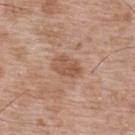notes=imaged on a skin check; not biopsied
image source=15 mm crop, total-body photography
patient=male, aged approximately 50
image-analysis metrics=an area of roughly 6 mm², an eccentricity of roughly 0.75, and a symmetry-axis asymmetry near 0.2; a lesion color around L≈54 a*≈21 b*≈31 in CIELAB; a border-irregularity rating of about 2/10 and radial color variation of about 1; a nevus-likeness score of about 0/100 and lesion-presence confidence of about 100/100
lesion size=≈3.5 mm
location=the upper back
tile lighting=white-light illumination